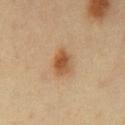Notes:
- notes · no biopsy performed (imaged during a skin exam)